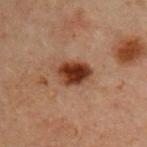This lesion was catalogued during total-body skin photography and was not selected for biopsy. A male subject, about 60 years old. A 15 mm crop from a total-body photograph taken for skin-cancer surveillance. From the chest. Captured under cross-polarized illumination. Measured at roughly 4 mm in maximum diameter.